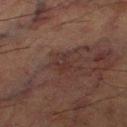| feature | finding |
|---|---|
| biopsy status | no biopsy performed (imaged during a skin exam) |
| size | ~2.5 mm (longest diameter) |
| patient | male, aged 63 to 67 |
| acquisition | ~15 mm crop, total-body skin-cancer survey |
| anatomic site | the left thigh |
| lighting | cross-polarized |